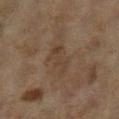Part of a total-body skin-imaging series; this lesion was reviewed on a skin check and was not flagged for biopsy.
The subject is a female aged approximately 60.
Measured at roughly 4 mm in maximum diameter.
A 15 mm crop from a total-body photograph taken for skin-cancer surveillance.
An algorithmic analysis of the crop reported a lesion area of about 7 mm² and two-axis asymmetry of about 0.35.
The lesion is on the left lower leg.